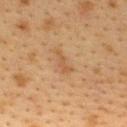Q: Was a biopsy performed?
A: imaged on a skin check; not biopsied
Q: What lighting was used for the tile?
A: cross-polarized
Q: What kind of image is this?
A: 15 mm crop, total-body photography
Q: Where on the body is the lesion?
A: the upper back
Q: Who is the patient?
A: female, aged around 40